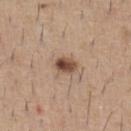Q: Was a biopsy performed?
A: imaged on a skin check; not biopsied
Q: Where on the body is the lesion?
A: the chest
Q: What kind of image is this?
A: ~15 mm tile from a whole-body skin photo
Q: What are the patient's age and sex?
A: male, aged approximately 60
Q: Illumination type?
A: white-light illumination